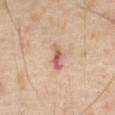<case>
  <biopsy_status>not biopsied; imaged during a skin examination</biopsy_status>
</case>